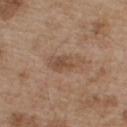Q: Was this lesion biopsied?
A: no biopsy performed (imaged during a skin exam)
Q: What lighting was used for the tile?
A: white-light illumination
Q: Where on the body is the lesion?
A: the chest
Q: What are the patient's age and sex?
A: male, approximately 55 years of age
Q: What is the imaging modality?
A: total-body-photography crop, ~15 mm field of view